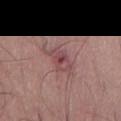Image and clinical context:
Longest diameter approximately 5 mm. The lesion is on the leg. The patient is a male aged around 50. A 15 mm close-up extracted from a 3D total-body photography capture.